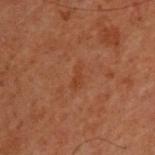Part of a total-body skin-imaging series; this lesion was reviewed on a skin check and was not flagged for biopsy. From the upper back. Captured under cross-polarized illumination. About 3 mm across. The total-body-photography lesion software estimated border irregularity of about 5 on a 0–10 scale, a color-variation rating of about 0.5/10, and a peripheral color-asymmetry measure near 0. It also reported a lesion-detection confidence of about 100/100. The patient is a male aged around 60. Cropped from a total-body skin-imaging series; the visible field is about 15 mm.